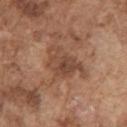Q: Was a biopsy performed?
A: no biopsy performed (imaged during a skin exam)